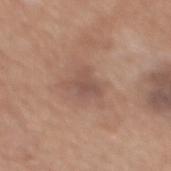Part of a total-body skin-imaging series; this lesion was reviewed on a skin check and was not flagged for biopsy. The subject is a female in their mid- to late 50s. Cropped from a whole-body photographic skin survey; the tile spans about 15 mm. Automated image analysis of the tile measured an area of roughly 4.5 mm², a shape eccentricity near 0.7, and two-axis asymmetry of about 0.35. It also reported a lesion color around L≈51 a*≈19 b*≈25 in CIELAB and about 7 CIELAB-L* units darker than the surrounding skin. And it measured a nevus-likeness score of about 0/100 and a detector confidence of about 100 out of 100 that the crop contains a lesion. Imaged with white-light lighting. Measured at roughly 3 mm in maximum diameter. On the mid back.